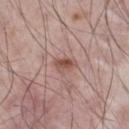The lesion was tiled from a total-body skin photograph and was not biopsied. The recorded lesion diameter is about 2.5 mm. A lesion tile, about 15 mm wide, cut from a 3D total-body photograph. On the chest. An algorithmic analysis of the crop reported a lesion area of about 3.5 mm². It also reported a mean CIELAB color near L≈51 a*≈22 b*≈25, roughly 11 lightness units darker than nearby skin, and a lesion-to-skin contrast of about 8 (normalized; higher = more distinct). The analysis additionally found a classifier nevus-likeness of about 70/100 and lesion-presence confidence of about 100/100. This is a white-light tile. The subject is a male in their mid- to late 60s.